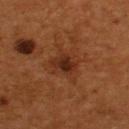This lesion was catalogued during total-body skin photography and was not selected for biopsy.
The lesion is located on the upper back.
This image is a 15 mm lesion crop taken from a total-body photograph.
A male subject, aged around 55.
Longest diameter approximately 3 mm.